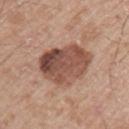{
  "biopsy_status": "not biopsied; imaged during a skin examination",
  "patient": {
    "sex": "male",
    "age_approx": 65
  },
  "image": {
    "source": "total-body photography crop",
    "field_of_view_mm": 15
  },
  "lighting": "white-light",
  "site": "arm"
}